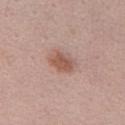Clinical impression:
The lesion was photographed on a routine skin check and not biopsied; there is no pathology result.